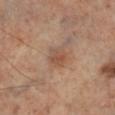biopsy status = no biopsy performed (imaged during a skin exam) | image source = ~15 mm crop, total-body skin-cancer survey | location = the right lower leg | size = ~2.5 mm (longest diameter) | tile lighting = cross-polarized | automated metrics = a lesion color around L≈51 a*≈20 b*≈30 in CIELAB, roughly 8 lightness units darker than nearby skin, and a normalized border contrast of about 6; internal color variation of about 2.5 on a 0–10 scale and radial color variation of about 1.